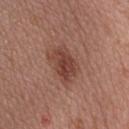lighting = white-light illumination | anatomic site = the upper back | imaging modality = total-body-photography crop, ~15 mm field of view | automated metrics = a border-irregularity rating of about 3/10, a color-variation rating of about 3.5/10, and peripheral color asymmetry of about 1 | lesion size = about 4.5 mm | subject = female, about 50 years old.